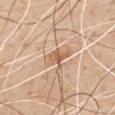Captured during whole-body skin photography for melanoma surveillance; the lesion was not biopsied. The subject is a male in their 50s. A lesion tile, about 15 mm wide, cut from a 3D total-body photograph. Automated image analysis of the tile measured a mean CIELAB color near L≈63 a*≈19 b*≈34, a lesion–skin lightness drop of about 9, and a normalized border contrast of about 6.5. The lesion is on the front of the torso.